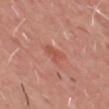notes = catalogued during a skin exam; not biopsied.